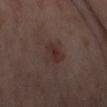The lesion was tiled from a total-body skin photograph and was not biopsied.
A female patient, aged 53 to 57.
Located on the leg.
This image is a 15 mm lesion crop taken from a total-body photograph.
Imaged with cross-polarized lighting.